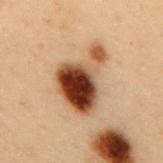Q: Was a biopsy performed?
A: no biopsy performed (imaged during a skin exam)
Q: Lesion size?
A: ~7 mm (longest diameter)
Q: Who is the patient?
A: male, roughly 55 years of age
Q: What kind of image is this?
A: total-body-photography crop, ~15 mm field of view
Q: What did automated image analysis measure?
A: a footprint of about 20 mm², an eccentricity of roughly 0.75, and a shape-asymmetry score of about 0.5 (0 = symmetric); an automated nevus-likeness rating near 100 out of 100 and lesion-presence confidence of about 100/100
Q: Lesion location?
A: the back
Q: How was the tile lit?
A: cross-polarized